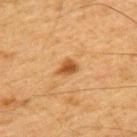Assessment: Captured during whole-body skin photography for melanoma surveillance; the lesion was not biopsied. Acquisition and patient details: A 15 mm crop from a total-body photograph taken for skin-cancer surveillance. The patient is a male aged 63–67. The tile uses cross-polarized illumination. The lesion is on the upper back. An algorithmic analysis of the crop reported an area of roughly 3.5 mm², a shape eccentricity near 0.75, and a shape-asymmetry score of about 0.3 (0 = symmetric). And it measured a nevus-likeness score of about 90/100 and lesion-presence confidence of about 100/100.